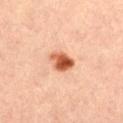Q: Is there a histopathology result?
A: imaged on a skin check; not biopsied
Q: Automated lesion metrics?
A: a lesion color around L≈57 a*≈29 b*≈38 in CIELAB and a lesion-to-skin contrast of about 11.5 (normalized; higher = more distinct); a nevus-likeness score of about 100/100
Q: What is the imaging modality?
A: ~15 mm crop, total-body skin-cancer survey
Q: How large is the lesion?
A: ≈3 mm
Q: What are the patient's age and sex?
A: female, roughly 45 years of age
Q: What lighting was used for the tile?
A: cross-polarized
Q: Lesion location?
A: the left thigh